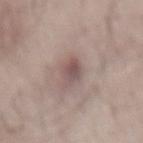The lesion was tiled from a total-body skin photograph and was not biopsied. Cropped from a total-body skin-imaging series; the visible field is about 15 mm. Imaged with white-light lighting. The recorded lesion diameter is about 3 mm. The lesion is on the back. The patient is a male in their 50s. An algorithmic analysis of the crop reported a lesion area of about 5 mm² and an outline eccentricity of about 0.8 (0 = round, 1 = elongated). It also reported a mean CIELAB color near L≈51 a*≈17 b*≈18, a lesion–skin lightness drop of about 10, and a normalized lesion–skin contrast near 7.5.